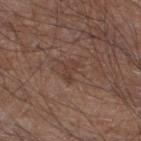The lesion was photographed on a routine skin check and not biopsied; there is no pathology result. The lesion is located on the left lower leg. A male subject in their mid-50s. A close-up tile cropped from a whole-body skin photograph, about 15 mm across.